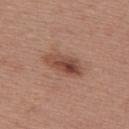This lesion was catalogued during total-body skin photography and was not selected for biopsy.